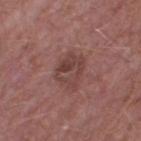The lesion was tiled from a total-body skin photograph and was not biopsied. Located on the right thigh. Captured under white-light illumination. The lesion-visualizer software estimated an outline eccentricity of about 0.6 (0 = round, 1 = elongated). And it measured a color-variation rating of about 5.5/10 and radial color variation of about 2.5. A male patient, aged 53–57. This image is a 15 mm lesion crop taken from a total-body photograph. About 4 mm across.